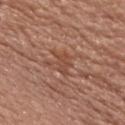The lesion was photographed on a routine skin check and not biopsied; there is no pathology result.
Approximately 3.5 mm at its widest.
A male subject roughly 55 years of age.
The lesion is located on the front of the torso.
A lesion tile, about 15 mm wide, cut from a 3D total-body photograph.
Automated tile analysis of the lesion measured a footprint of about 5 mm², an eccentricity of roughly 0.8, and two-axis asymmetry of about 0.6.
Imaged with white-light lighting.